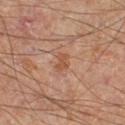  biopsy_status: not biopsied; imaged during a skin examination
  lesion_size:
    long_diameter_mm_approx: 2.5
  patient:
    sex: male
    age_approx: 70
  site: left lower leg
  automated_metrics:
    area_mm2_approx: 3.5
    eccentricity: 0.75
    shape_asymmetry: 0.3
    nevus_likeness_0_100: 0
  image:
    source: total-body photography crop
    field_of_view_mm: 15
  lighting: cross-polarized